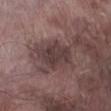Q: Was a biopsy performed?
A: no biopsy performed (imaged during a skin exam)
Q: Illumination type?
A: white-light illumination
Q: How large is the lesion?
A: about 4.5 mm
Q: What kind of image is this?
A: ~15 mm crop, total-body skin-cancer survey
Q: Automated lesion metrics?
A: a footprint of about 11 mm², a shape eccentricity near 0.8, and two-axis asymmetry of about 0.25; an average lesion color of about L≈37 a*≈16 b*≈15 (CIELAB); border irregularity of about 3 on a 0–10 scale and a color-variation rating of about 2.5/10
Q: Patient demographics?
A: male, aged around 75
Q: What is the anatomic site?
A: the right lower leg